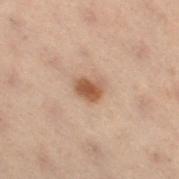Notes:
– workup: catalogued during a skin exam; not biopsied
– site: the left leg
– lighting: cross-polarized illumination
– lesion size: ≈2.5 mm
– subject: female, in their mid-50s
– automated lesion analysis: a footprint of about 4.5 mm² and a symmetry-axis asymmetry near 0.25; a mean CIELAB color near L≈45 a*≈18 b*≈28, a lesion–skin lightness drop of about 11, and a lesion-to-skin contrast of about 9 (normalized; higher = more distinct)
– image: 15 mm crop, total-body photography